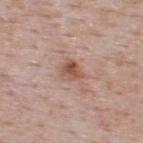Part of a total-body skin-imaging series; this lesion was reviewed on a skin check and was not flagged for biopsy. The lesion-visualizer software estimated an average lesion color of about L≈54 a*≈20 b*≈28 (CIELAB), roughly 10 lightness units darker than nearby skin, and a normalized border contrast of about 7.5. And it measured internal color variation of about 5.5 on a 0–10 scale. Approximately 3 mm at its widest. Imaged with white-light lighting. The lesion is on the upper back. A roughly 15 mm field-of-view crop from a total-body skin photograph. The patient is a male in their 50s.